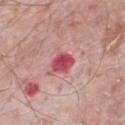Image and clinical context: A 15 mm close-up extracted from a 3D total-body photography capture. On the front of the torso. Automated image analysis of the tile measured a lesion area of about 5.5 mm² and a symmetry-axis asymmetry near 0.15. The software also gave a border-irregularity index near 1.5/10, a color-variation rating of about 3.5/10, and peripheral color asymmetry of about 1. The analysis additionally found lesion-presence confidence of about 100/100. The subject is a male aged around 70. The tile uses white-light illumination.